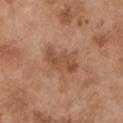No biopsy was performed on this lesion — it was imaged during a full skin examination and was not determined to be concerning. A roughly 15 mm field-of-view crop from a total-body skin photograph. The subject is a male in their mid- to late 50s. The lesion's longest dimension is about 4.5 mm. Imaged with white-light lighting. Located on the left upper arm.